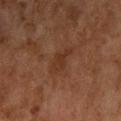{"biopsy_status": "not biopsied; imaged during a skin examination", "patient": {"sex": "female", "age_approx": 60}, "site": "left forearm", "lesion_size": {"long_diameter_mm_approx": 3.5}, "lighting": "cross-polarized", "image": {"source": "total-body photography crop", "field_of_view_mm": 15}}